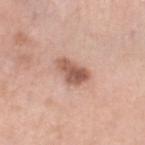Q: Was a biopsy performed?
A: imaged on a skin check; not biopsied
Q: Illumination type?
A: white-light illumination
Q: Who is the patient?
A: female, approximately 40 years of age
Q: How was this image acquired?
A: 15 mm crop, total-body photography
Q: Lesion location?
A: the left lower leg
Q: How large is the lesion?
A: ~4 mm (longest diameter)